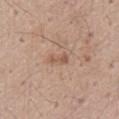acquisition=~15 mm crop, total-body skin-cancer survey | patient=male, aged approximately 40 | anatomic site=the lower back | lighting=white-light.